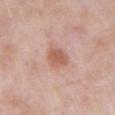{
  "biopsy_status": "not biopsied; imaged during a skin examination",
  "lesion_size": {
    "long_diameter_mm_approx": 3.0
  },
  "image": {
    "source": "total-body photography crop",
    "field_of_view_mm": 15
  },
  "lighting": "white-light",
  "patient": {
    "sex": "male",
    "age_approx": 55
  },
  "automated_metrics": {
    "cielab_L": 59,
    "cielab_a": 22,
    "cielab_b": 29,
    "vs_skin_darker_L": 10.0
  },
  "site": "abdomen"
}